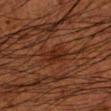Context:
An algorithmic analysis of the crop reported an eccentricity of roughly 0.8. The analysis additionally found a normalized lesion–skin contrast near 6.5. It also reported border irregularity of about 3.5 on a 0–10 scale and a peripheral color-asymmetry measure near 1. Captured under cross-polarized illumination. This image is a 15 mm lesion crop taken from a total-body photograph. A male patient, aged around 50. On the left forearm.